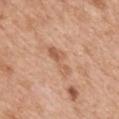Assessment: Imaged during a routine full-body skin examination; the lesion was not biopsied and no histopathology is available. Clinical summary: The lesion is on the back. The patient is a female about 40 years old. An algorithmic analysis of the crop reported an average lesion color of about L≈59 a*≈22 b*≈33 (CIELAB). The software also gave a border-irregularity index near 5.5/10 and a within-lesion color-variation index near 3/10. A roughly 15 mm field-of-view crop from a total-body skin photograph. The tile uses white-light illumination.